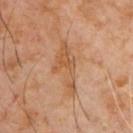Captured during whole-body skin photography for melanoma surveillance; the lesion was not biopsied.
The subject is a male aged 58 to 62.
A lesion tile, about 15 mm wide, cut from a 3D total-body photograph.
Imaged with cross-polarized lighting.
On the front of the torso.
Approximately 6.5 mm at its widest.